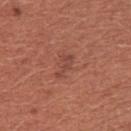Assessment: The lesion was tiled from a total-body skin photograph and was not biopsied. Acquisition and patient details: The tile uses white-light illumination. An algorithmic analysis of the crop reported a shape-asymmetry score of about 0.5 (0 = symmetric). The software also gave a nevus-likeness score of about 0/100. This image is a 15 mm lesion crop taken from a total-body photograph. The recorded lesion diameter is about 3 mm. The lesion is located on the chest. A female patient, roughly 35 years of age.